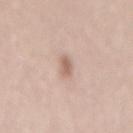biopsy status: imaged on a skin check; not biopsied | location: the back | automated metrics: an eccentricity of roughly 0.85 and a shape-asymmetry score of about 0.3 (0 = symmetric); roughly 11 lightness units darker than nearby skin and a lesion-to-skin contrast of about 7 (normalized; higher = more distinct); a lesion-detection confidence of about 100/100 | subject: male, aged around 45 | diameter: about 2.5 mm | image: ~15 mm tile from a whole-body skin photo | lighting: white-light illumination.